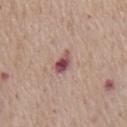Part of a total-body skin-imaging series; this lesion was reviewed on a skin check and was not flagged for biopsy. A male patient in their mid- to late 70s. The lesion is on the chest. About 3 mm across. Imaged with white-light lighting. A region of skin cropped from a whole-body photographic capture, roughly 15 mm wide.